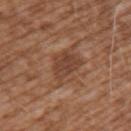notes: imaged on a skin check; not biopsied | site: the left upper arm | illumination: white-light illumination | patient: male, aged around 75 | image: ~15 mm crop, total-body skin-cancer survey | size: about 4.5 mm.